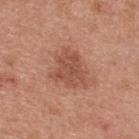Impression: This lesion was catalogued during total-body skin photography and was not selected for biopsy. Image and clinical context: The lesion-visualizer software estimated border irregularity of about 4.5 on a 0–10 scale, internal color variation of about 2.5 on a 0–10 scale, and radial color variation of about 1. It also reported a classifier nevus-likeness of about 15/100. A 15 mm crop from a total-body photograph taken for skin-cancer surveillance. This is a white-light tile. From the upper back. A male subject about 30 years old.